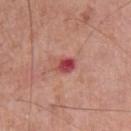On the chest.
A male subject, aged 73 to 77.
The tile uses white-light illumination.
Measured at roughly 2.5 mm in maximum diameter.
A region of skin cropped from a whole-body photographic capture, roughly 15 mm wide.
Automated tile analysis of the lesion measured a mean CIELAB color near L≈48 a*≈34 b*≈25, a lesion–skin lightness drop of about 13, and a normalized lesion–skin contrast near 9.5. The analysis additionally found a classifier nevus-likeness of about 0/100 and a detector confidence of about 100 out of 100 that the crop contains a lesion.